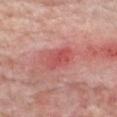Clinical impression:
No biopsy was performed on this lesion — it was imaged during a full skin examination and was not determined to be concerning.
Acquisition and patient details:
A 15 mm crop from a total-body photograph taken for skin-cancer surveillance. A male subject, approximately 75 years of age. This is a white-light tile. Approximately 3.5 mm at its widest. The lesion is located on the right forearm.